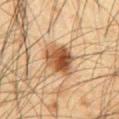Clinical summary: An algorithmic analysis of the crop reported a lesion area of about 12 mm². The software also gave a lesion color around L≈44 a*≈19 b*≈32 in CIELAB, roughly 14 lightness units darker than nearby skin, and a lesion-to-skin contrast of about 10.5 (normalized; higher = more distinct). The analysis additionally found a border-irregularity rating of about 2/10. The tile uses cross-polarized illumination. The patient is a male aged around 65. A lesion tile, about 15 mm wide, cut from a 3D total-body photograph. Approximately 4.5 mm at its widest. The lesion is on the abdomen.